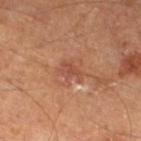Part of a total-body skin-imaging series; this lesion was reviewed on a skin check and was not flagged for biopsy.
On the left lower leg.
A 15 mm close-up tile from a total-body photography series done for melanoma screening.
The patient is a male approximately 65 years of age.
Automated tile analysis of the lesion measured a lesion color around L≈47 a*≈25 b*≈30 in CIELAB, about 7 CIELAB-L* units darker than the surrounding skin, and a lesion-to-skin contrast of about 5.5 (normalized; higher = more distinct). It also reported a classifier nevus-likeness of about 5/100 and a lesion-detection confidence of about 100/100.
Captured under cross-polarized illumination.
Longest diameter approximately 2.5 mm.